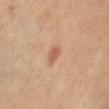workup: no biopsy performed (imaged during a skin exam)
patient: female, in their mid-60s
image source: total-body-photography crop, ~15 mm field of view
lesion size: ~2.5 mm (longest diameter)
lighting: cross-polarized
site: the left leg